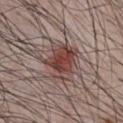Clinical impression:
This lesion was catalogued during total-body skin photography and was not selected for biopsy.
Background:
The patient is a male aged around 40. This is a white-light tile. The lesion is on the chest. Measured at roughly 4.5 mm in maximum diameter. A roughly 15 mm field-of-view crop from a total-body skin photograph.